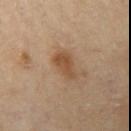Part of a total-body skin-imaging series; this lesion was reviewed on a skin check and was not flagged for biopsy. The recorded lesion diameter is about 4 mm. Cropped from a whole-body photographic skin survey; the tile spans about 15 mm. Automated image analysis of the tile measured an area of roughly 7.5 mm², a shape eccentricity near 0.8, and a shape-asymmetry score of about 0.25 (0 = symmetric). It also reported a lesion color around L≈48 a*≈18 b*≈32 in CIELAB and roughly 9 lightness units darker than nearby skin. It also reported a border-irregularity index near 2.5/10, internal color variation of about 3 on a 0–10 scale, and a peripheral color-asymmetry measure near 1. Located on the left upper arm. Captured under cross-polarized illumination. A male subject aged around 85 or older.